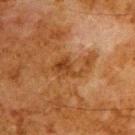| field | value |
|---|---|
| size | ≈6.5 mm |
| location | the upper back |
| automated lesion analysis | an average lesion color of about L≈35 a*≈20 b*≈33 (CIELAB), a lesion–skin lightness drop of about 7, and a normalized lesion–skin contrast near 6.5; a color-variation rating of about 4/10; a classifier nevus-likeness of about 0/100 and a lesion-detection confidence of about 100/100 |
| subject | male, aged 78 to 82 |
| lighting | cross-polarized |
| image source | total-body-photography crop, ~15 mm field of view |